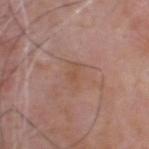{"biopsy_status": "not biopsied; imaged during a skin examination", "site": "head or neck", "lighting": "white-light", "image": {"source": "total-body photography crop", "field_of_view_mm": 15}, "automated_metrics": {"eccentricity": 0.85, "shape_asymmetry": 0.45, "nevus_likeness_0_100": 0}, "lesion_size": {"long_diameter_mm_approx": 2.5}, "patient": {"sex": "male", "age_approx": 65}}